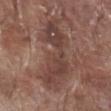A 15 mm close-up tile from a total-body photography series done for melanoma screening. The lesion is on the right lower leg. The subject is a male in their 70s.